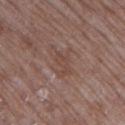<record>
  <biopsy_status>not biopsied; imaged during a skin examination</biopsy_status>
  <site>right upper arm</site>
  <automated_metrics>
    <cielab_L>44</cielab_L>
    <cielab_a>18</cielab_a>
    <cielab_b>24</cielab_b>
    <vs_skin_darker_L>6.0</vs_skin_darker_L>
    <vs_skin_contrast_norm>5.0</vs_skin_contrast_norm>
    <nevus_likeness_0_100>0</nevus_likeness_0_100>
    <lesion_detection_confidence_0_100>95</lesion_detection_confidence_0_100>
  </automated_metrics>
  <image>
    <source>total-body photography crop</source>
    <field_of_view_mm>15</field_of_view_mm>
  </image>
  <patient>
    <sex>female</sex>
    <age_approx>70</age_approx>
  </patient>
  <lighting>white-light</lighting>
</record>